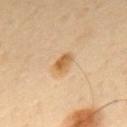  biopsy_status: not biopsied; imaged during a skin examination
  patient:
    sex: male
    age_approx: 60
  image:
    source: total-body photography crop
    field_of_view_mm: 15
  site: mid back